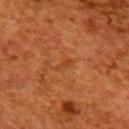{
  "biopsy_status": "not biopsied; imaged during a skin examination",
  "site": "upper back",
  "patient": {
    "sex": "female",
    "age_approx": 50
  },
  "image": {
    "source": "total-body photography crop",
    "field_of_view_mm": 15
  }
}